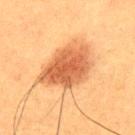The lesion was tiled from a total-body skin photograph and was not biopsied. Cropped from a whole-body photographic skin survey; the tile spans about 15 mm. The lesion is located on the upper back. The lesion-visualizer software estimated an eccentricity of roughly 0.75. And it measured an average lesion color of about L≈48 a*≈24 b*≈35 (CIELAB), roughly 13 lightness units darker than nearby skin, and a normalized lesion–skin contrast near 9. About 7 mm across. Imaged with cross-polarized lighting. A male patient aged approximately 50.